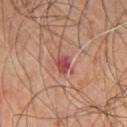{
  "biopsy_status": "not biopsied; imaged during a skin examination",
  "lesion_size": {
    "long_diameter_mm_approx": 2.5
  },
  "patient": {
    "sex": "male",
    "age_approx": 70
  },
  "site": "chest",
  "lighting": "cross-polarized",
  "image": {
    "source": "total-body photography crop",
    "field_of_view_mm": 15
  },
  "automated_metrics": {
    "border_irregularity_0_10": 2.0,
    "color_variation_0_10": 4.5,
    "peripheral_color_asymmetry": 1.5
  }
}